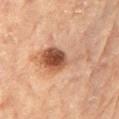follow-up: catalogued during a skin exam; not biopsied | body site: the right arm | acquisition: total-body-photography crop, ~15 mm field of view | subject: female, in their 80s | illumination: cross-polarized illumination | automated metrics: a mean CIELAB color near L≈50 a*≈21 b*≈30, about 13 CIELAB-L* units darker than the surrounding skin, and a normalized border contrast of about 9 | diameter: about 7 mm.